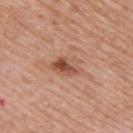Impression: This lesion was catalogued during total-body skin photography and was not selected for biopsy.